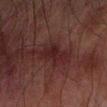The lesion was photographed on a routine skin check and not biopsied; there is no pathology result. From the right forearm. Automated tile analysis of the lesion measured an outline eccentricity of about 0.9 (0 = round, 1 = elongated) and a shape-asymmetry score of about 0.3 (0 = symmetric). It also reported a lesion color around L≈17 a*≈18 b*≈15 in CIELAB, about 6 CIELAB-L* units darker than the surrounding skin, and a lesion-to-skin contrast of about 8 (normalized; higher = more distinct). The software also gave a within-lesion color-variation index near 2/10 and radial color variation of about 0.5. This is a cross-polarized tile. A male subject, roughly 65 years of age. Approximately 4.5 mm at its widest. A lesion tile, about 15 mm wide, cut from a 3D total-body photograph.